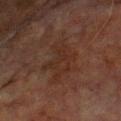Recorded during total-body skin imaging; not selected for excision or biopsy.
The lesion is located on the front of the torso.
A male patient, about 75 years old.
A 15 mm crop from a total-body photograph taken for skin-cancer surveillance.